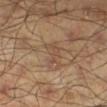follow-up: no biopsy performed (imaged during a skin exam)
subject: male, roughly 45 years of age
image: ~15 mm crop, total-body skin-cancer survey
location: the right lower leg